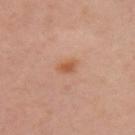Case summary:
* biopsy status · no biopsy performed (imaged during a skin exam)
* subject · female, approximately 40 years of age
* automated metrics · a lesion area of about 3.5 mm² and a symmetry-axis asymmetry near 0.25; a lesion color around L≈56 a*≈24 b*≈35 in CIELAB, about 9 CIELAB-L* units darker than the surrounding skin, and a normalized lesion–skin contrast near 7.5; a border-irregularity index near 2.5/10, a within-lesion color-variation index near 1/10, and peripheral color asymmetry of about 0.5
* location · the arm
* diameter · about 2.5 mm
* tile lighting · cross-polarized illumination
* imaging modality · ~15 mm tile from a whole-body skin photo